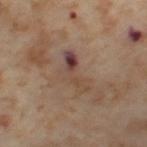{"biopsy_status": "not biopsied; imaged during a skin examination", "lighting": "cross-polarized", "lesion_size": {"long_diameter_mm_approx": 5.0}, "patient": {"sex": "female", "age_approx": 55}, "image": {"source": "total-body photography crop", "field_of_view_mm": 15}, "site": "left thigh"}